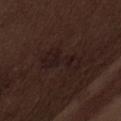subject: male, approximately 70 years of age | body site: the abdomen | image source: ~15 mm tile from a whole-body skin photo | lesion size: ~5 mm (longest diameter) | automated lesion analysis: an area of roughly 9 mm² and a symmetry-axis asymmetry near 0.4 | lighting: white-light.